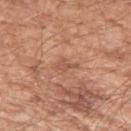<record>
<biopsy_status>not biopsied; imaged during a skin examination</biopsy_status>
<site>arm</site>
<lesion_size>
  <long_diameter_mm_approx>2.5</long_diameter_mm_approx>
</lesion_size>
<image>
  <source>total-body photography crop</source>
  <field_of_view_mm>15</field_of_view_mm>
</image>
<lighting>white-light</lighting>
<automated_metrics>
  <area_mm2_approx>3.0</area_mm2_approx>
  <eccentricity>0.85</eccentricity>
  <border_irregularity_0_10>5.5</border_irregularity_0_10>
  <color_variation_0_10>0.0</color_variation_0_10>
  <peripheral_color_asymmetry>0.0</peripheral_color_asymmetry>
  <nevus_likeness_0_100>0</nevus_likeness_0_100>
  <lesion_detection_confidence_0_100>90</lesion_detection_confidence_0_100>
</automated_metrics>
<patient>
  <sex>male</sex>
  <age_approx>65</age_approx>
</patient>
</record>